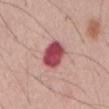Findings:
• biopsy status: total-body-photography surveillance lesion; no biopsy
• size: ≈4 mm
• lighting: white-light illumination
• patient: male, in their mid-50s
• imaging modality: total-body-photography crop, ~15 mm field of view